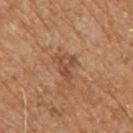workup: total-body-photography surveillance lesion; no biopsy
site: the chest
subject: male, aged 68–72
imaging modality: ~15 mm tile from a whole-body skin photo